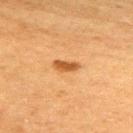biopsy status: imaged on a skin check; not biopsied | TBP lesion metrics: an area of roughly 3 mm², an eccentricity of roughly 0.9, and a symmetry-axis asymmetry near 0.3; an average lesion color of about L≈47 a*≈24 b*≈40 (CIELAB), a lesion–skin lightness drop of about 12, and a lesion-to-skin contrast of about 9 (normalized; higher = more distinct) | diameter: about 3 mm | image: ~15 mm crop, total-body skin-cancer survey | lighting: cross-polarized illumination | subject: female, approximately 50 years of age | anatomic site: the right upper arm.